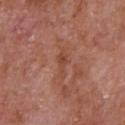Background:
Approximately 3.5 mm at its widest. A male patient aged 63 to 67. A roughly 15 mm field-of-view crop from a total-body skin photograph. On the chest.